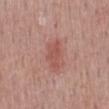The lesion was photographed on a routine skin check and not biopsied; there is no pathology result. A 15 mm close-up extracted from a 3D total-body photography capture. Automated image analysis of the tile measured an average lesion color of about L≈53 a*≈26 b*≈26 (CIELAB), about 8 CIELAB-L* units darker than the surrounding skin, and a normalized border contrast of about 5.5. The analysis additionally found a border-irregularity index near 3.5/10, a within-lesion color-variation index near 1.5/10, and radial color variation of about 0.5. From the mid back. A male subject in their 50s. Measured at roughly 3.5 mm in maximum diameter.